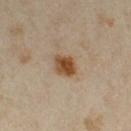Recorded during total-body skin imaging; not selected for excision or biopsy.
The lesion-visualizer software estimated an average lesion color of about L≈45 a*≈18 b*≈33 (CIELAB), roughly 13 lightness units darker than nearby skin, and a normalized lesion–skin contrast near 11. The analysis additionally found a classifier nevus-likeness of about 100/100 and a lesion-detection confidence of about 100/100.
This is a cross-polarized tile.
A 15 mm close-up extracted from a 3D total-body photography capture.
A female patient about 35 years old.
Located on the right thigh.
Approximately 3 mm at its widest.